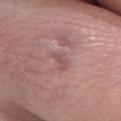Part of a total-body skin-imaging series; this lesion was reviewed on a skin check and was not flagged for biopsy. Captured under white-light illumination. A lesion tile, about 15 mm wide, cut from a 3D total-body photograph. The lesion's longest dimension is about 3 mm. A male patient, approximately 30 years of age.